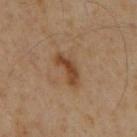Clinical impression: Imaged during a routine full-body skin examination; the lesion was not biopsied and no histopathology is available. Context: A male patient, aged 58–62. On the back. A region of skin cropped from a whole-body photographic capture, roughly 15 mm wide. The total-body-photography lesion software estimated an area of roughly 5.5 mm² and a symmetry-axis asymmetry near 0.45. It also reported roughly 10 lightness units darker than nearby skin. It also reported a border-irregularity index near 5/10, a color-variation rating of about 2.5/10, and a peripheral color-asymmetry measure near 0.5. Approximately 4.5 mm at its widest.